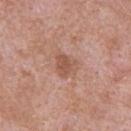A lesion tile, about 15 mm wide, cut from a 3D total-body photograph. From the upper back. The patient is a male aged 48–52.